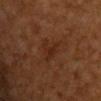| key | value |
|---|---|
| tile lighting | cross-polarized illumination |
| acquisition | 15 mm crop, total-body photography |
| patient | male, aged 58 to 62 |
| anatomic site | the chest |